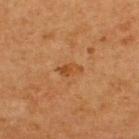Notes:
- site: the upper back
- automated lesion analysis: a nevus-likeness score of about 0/100 and a lesion-detection confidence of about 100/100
- image source: total-body-photography crop, ~15 mm field of view
- patient: male, in their mid- to late 60s